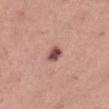Clinical impression: Captured during whole-body skin photography for melanoma surveillance; the lesion was not biopsied. Background: A female patient roughly 40 years of age. From the leg. Longest diameter approximately 2 mm. A region of skin cropped from a whole-body photographic capture, roughly 15 mm wide.